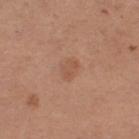biopsy_status: not biopsied; imaged during a skin examination
automated_metrics:
  vs_skin_contrast_norm: 4.5
  color_variation_0_10: 1.5
  peripheral_color_asymmetry: 0.5
  nevus_likeness_0_100: 15
lighting: white-light
site: left thigh
image:
  source: total-body photography crop
  field_of_view_mm: 15
patient:
  sex: female
  age_approx: 30
lesion_size:
  long_diameter_mm_approx: 2.5